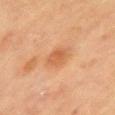image — ~15 mm crop, total-body skin-cancer survey
anatomic site — the left upper arm
diameter — about 2.5 mm
subject — female, aged 78 to 82
TBP lesion metrics — a footprint of about 4.5 mm², an outline eccentricity of about 0.5 (0 = round, 1 = elongated), and a symmetry-axis asymmetry near 0.2; a border-irregularity rating of about 2/10 and radial color variation of about 1